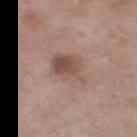Q: Was this lesion biopsied?
A: imaged on a skin check; not biopsied
Q: How was the tile lit?
A: white-light illumination
Q: What kind of image is this?
A: total-body-photography crop, ~15 mm field of view
Q: How large is the lesion?
A: about 5.5 mm
Q: What are the patient's age and sex?
A: female, aged 53–57
Q: What is the anatomic site?
A: the right thigh